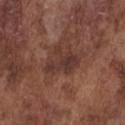This lesion was catalogued during total-body skin photography and was not selected for biopsy.
A 15 mm close-up tile from a total-body photography series done for melanoma screening.
The patient is a male aged 73 to 77.
The lesion is located on the chest.